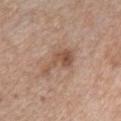Captured under white-light illumination. A close-up tile cropped from a whole-body skin photograph, about 15 mm across. On the chest. The subject is a female about 40 years old.